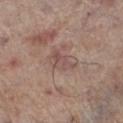notes: imaged on a skin check; not biopsied | image source: total-body-photography crop, ~15 mm field of view | site: the left lower leg | patient: male, approximately 70 years of age.